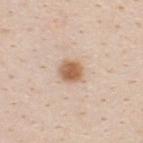The lesion was photographed on a routine skin check and not biopsied; there is no pathology result.
The subject is a male roughly 35 years of age.
Captured under white-light illumination.
A roughly 15 mm field-of-view crop from a total-body skin photograph.
Longest diameter approximately 3 mm.
The lesion is on the upper back.